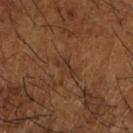Findings:
– subject: male, aged 63 to 67
– diameter: about 2.5 mm
– TBP lesion metrics: an area of roughly 2.5 mm², a shape eccentricity near 0.9, and two-axis asymmetry of about 0.5; an automated nevus-likeness rating near 0 out of 100 and lesion-presence confidence of about 70/100
– location: the right forearm
– image: ~15 mm tile from a whole-body skin photo